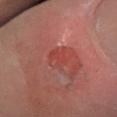{"biopsy_status": "not biopsied; imaged during a skin examination", "site": "leg", "automated_metrics": {"border_irregularity_0_10": 2.5, "color_variation_0_10": 3.0, "nevus_likeness_0_100": 5, "lesion_detection_confidence_0_100": 65}, "patient": {"sex": "female", "age_approx": 55}, "image": {"source": "total-body photography crop", "field_of_view_mm": 15}, "lighting": "cross-polarized"}